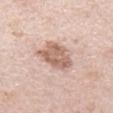follow-up = no biopsy performed (imaged during a skin exam); image source = total-body-photography crop, ~15 mm field of view; size = ~4.5 mm (longest diameter); lighting = white-light; location = the arm; subject = male, approximately 40 years of age.